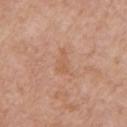This lesion was catalogued during total-body skin photography and was not selected for biopsy.
Cropped from a total-body skin-imaging series; the visible field is about 15 mm.
A male subject in their 80s.
From the chest.